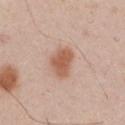Recorded during total-body skin imaging; not selected for excision or biopsy.
This image is a 15 mm lesion crop taken from a total-body photograph.
Located on the chest.
The lesion's longest dimension is about 4 mm.
A male patient about 60 years old.
This is a white-light tile.